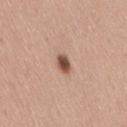{"biopsy_status": "not biopsied; imaged during a skin examination", "image": {"source": "total-body photography crop", "field_of_view_mm": 15}, "lesion_size": {"long_diameter_mm_approx": 2.5}, "lighting": "white-light", "patient": {"sex": "female", "age_approx": 30}, "site": "lower back"}